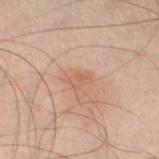Assessment: Imaged during a routine full-body skin examination; the lesion was not biopsied and no histopathology is available. Clinical summary: The lesion is on the left thigh. The tile uses cross-polarized illumination. Cropped from a whole-body photographic skin survey; the tile spans about 15 mm. A male patient, aged around 50.